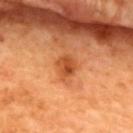{"biopsy_status": "not biopsied; imaged during a skin examination"}